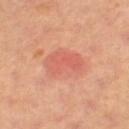Q: Is there a histopathology result?
A: total-body-photography surveillance lesion; no biopsy
Q: What is the imaging modality?
A: 15 mm crop, total-body photography
Q: What is the anatomic site?
A: the left thigh
Q: What are the patient's age and sex?
A: female, aged 63 to 67